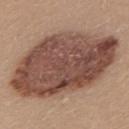subject = female, approximately 25 years of age; anatomic site = the upper back; diameter = about 13.5 mm; acquisition = ~15 mm tile from a whole-body skin photo.